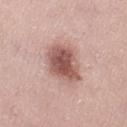follow-up — total-body-photography surveillance lesion; no biopsy | body site — the left thigh | illumination — white-light | image source — 15 mm crop, total-body photography | subject — female, about 40 years old.